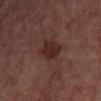Assessment:
This lesion was catalogued during total-body skin photography and was not selected for biopsy.
Clinical summary:
From the leg. A close-up tile cropped from a whole-body skin photograph, about 15 mm across. The patient is a female aged around 60. The lesion's longest dimension is about 4 mm. An algorithmic analysis of the crop reported a lesion area of about 7.5 mm², a shape eccentricity near 0.75, and a symmetry-axis asymmetry near 0.2. The software also gave a border-irregularity index near 2.5/10, internal color variation of about 2 on a 0–10 scale, and a peripheral color-asymmetry measure near 1. The software also gave an automated nevus-likeness rating near 50 out of 100.